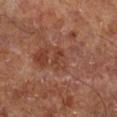Notes:
- notes · no biopsy performed (imaged during a skin exam)
- patient · in their mid-60s
- tile lighting · cross-polarized
- imaging modality · ~15 mm tile from a whole-body skin photo
- diameter · ~2.5 mm (longest diameter)
- automated lesion analysis · an area of roughly 3.5 mm² and a symmetry-axis asymmetry near 0.5
- site · the right lower leg